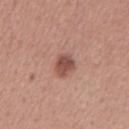  biopsy_status: not biopsied; imaged during a skin examination
  lesion_size:
    long_diameter_mm_approx: 3.0
  image:
    source: total-body photography crop
    field_of_view_mm: 15
  site: chest
  patient:
    sex: male
    age_approx: 45
  lighting: white-light